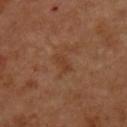biopsy_status: not biopsied; imaged during a skin examination
image:
  source: total-body photography crop
  field_of_view_mm: 15
patient:
  sex: male
  age_approx: 50
site: chest